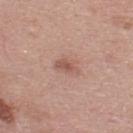{
  "biopsy_status": "not biopsied; imaged during a skin examination",
  "patient": {
    "sex": "male",
    "age_approx": 25
  },
  "lighting": "white-light",
  "lesion_size": {
    "long_diameter_mm_approx": 3.0
  },
  "image": {
    "source": "total-body photography crop",
    "field_of_view_mm": 15
  },
  "site": "back"
}